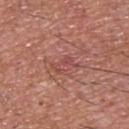Notes:
– diameter — ~3.5 mm (longest diameter)
– image source — 15 mm crop, total-body photography
– location — the upper back
– patient — male, aged 53 to 57